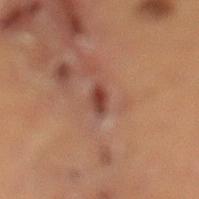Notes:
– tile lighting · cross-polarized
– subject · male, roughly 60 years of age
– size · about 3 mm
– anatomic site · the left lower leg
– image source · 15 mm crop, total-body photography